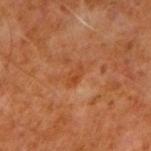No biopsy was performed on this lesion — it was imaged during a full skin examination and was not determined to be concerning. From the right upper arm. A male patient approximately 60 years of age. This is a cross-polarized tile. Longest diameter approximately 2.5 mm. A 15 mm crop from a total-body photograph taken for skin-cancer surveillance.